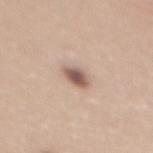Assessment: Part of a total-body skin-imaging series; this lesion was reviewed on a skin check and was not flagged for biopsy. Clinical summary: Captured under white-light illumination. A female subject in their mid- to late 30s. From the mid back. A lesion tile, about 15 mm wide, cut from a 3D total-body photograph.